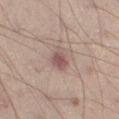Captured under cross-polarized illumination. The patient is a male roughly 50 years of age. The lesion is located on the left thigh. Automated tile analysis of the lesion measured a lesion area of about 5 mm² and a shape-asymmetry score of about 0.3 (0 = symmetric). About 3 mm across. This image is a 15 mm lesion crop taken from a total-body photograph.